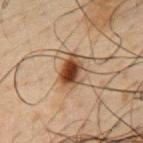Recorded during total-body skin imaging; not selected for excision or biopsy. The lesion is located on the mid back. The subject is a male aged approximately 50. The tile uses cross-polarized illumination. A roughly 15 mm field-of-view crop from a total-body skin photograph.